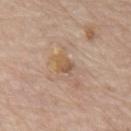biopsy_status: not biopsied; imaged during a skin examination
image:
  source: total-body photography crop
  field_of_view_mm: 15
lesion_size:
  long_diameter_mm_approx: 3.0
patient:
  sex: male
  age_approx: 80
site: chest
automated_metrics:
  border_irregularity_0_10: 3.5
  color_variation_0_10: 4.0
  peripheral_color_asymmetry: 1.0